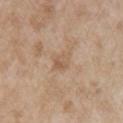Q: Was this lesion biopsied?
A: catalogued during a skin exam; not biopsied
Q: What is the anatomic site?
A: the right upper arm
Q: How was this image acquired?
A: ~15 mm crop, total-body skin-cancer survey
Q: What is the lesion's diameter?
A: about 3 mm
Q: Patient demographics?
A: male, in their mid- to late 60s
Q: What did automated image analysis measure?
A: an area of roughly 4.5 mm², an outline eccentricity of about 0.65 (0 = round, 1 = elongated), and two-axis asymmetry of about 0.25